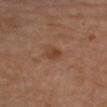Case summary:
- biopsy status · imaged on a skin check; not biopsied
- automated metrics · a lesion color around L≈43 a*≈21 b*≈31 in CIELAB and roughly 7 lightness units darker than nearby skin
- location · the right upper arm
- patient · female, about 65 years old
- acquisition · ~15 mm tile from a whole-body skin photo